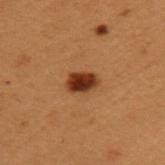Recorded during total-body skin imaging; not selected for excision or biopsy. This is a cross-polarized tile. The total-body-photography lesion software estimated a lesion area of about 5 mm², an outline eccentricity of about 0.7 (0 = round, 1 = elongated), and two-axis asymmetry of about 0.25. The software also gave a classifier nevus-likeness of about 100/100 and a lesion-detection confidence of about 100/100. The lesion is located on the left upper arm. A 15 mm close-up tile from a total-body photography series done for melanoma screening. A male subject approximately 50 years of age.